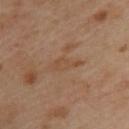Impression: This lesion was catalogued during total-body skin photography and was not selected for biopsy. Background: Measured at roughly 4 mm in maximum diameter. Captured under cross-polarized illumination. The lesion is located on the upper back. A female patient approximately 40 years of age. Cropped from a whole-body photographic skin survey; the tile spans about 15 mm. The total-body-photography lesion software estimated a mean CIELAB color near L≈49 a*≈18 b*≈32, roughly 5 lightness units darker than nearby skin, and a normalized lesion–skin contrast near 5. It also reported internal color variation of about 1 on a 0–10 scale and radial color variation of about 0.5.